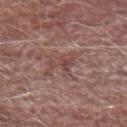* size: ≈3 mm
* image: 15 mm crop, total-body photography
* anatomic site: the right forearm
* subject: male, aged approximately 65